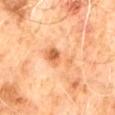Imaged during a routine full-body skin examination; the lesion was not biopsied and no histopathology is available. The lesion is located on the abdomen. Cropped from a total-body skin-imaging series; the visible field is about 15 mm. The subject is a male aged 58 to 62.